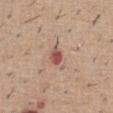No biopsy was performed on this lesion — it was imaged during a full skin examination and was not determined to be concerning.
About 2.5 mm across.
On the abdomen.
The tile uses white-light illumination.
A male patient, in their 60s.
A close-up tile cropped from a whole-body skin photograph, about 15 mm across.